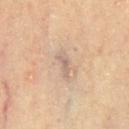The lesion was photographed on a routine skin check and not biopsied; there is no pathology result. Automated tile analysis of the lesion measured a mean CIELAB color near L≈62 a*≈15 b*≈26, about 8 CIELAB-L* units darker than the surrounding skin, and a lesion-to-skin contrast of about 6 (normalized; higher = more distinct). And it measured a classifier nevus-likeness of about 0/100 and lesion-presence confidence of about 60/100. This is a cross-polarized tile. A male subject aged 63 to 67. Cropped from a total-body skin-imaging series; the visible field is about 15 mm. On the chest.